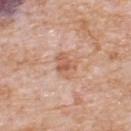Recorded during total-body skin imaging; not selected for excision or biopsy.
A male subject about 80 years old.
The lesion is on the upper back.
The recorded lesion diameter is about 3 mm.
This image is a 15 mm lesion crop taken from a total-body photograph.
The lesion-visualizer software estimated a shape-asymmetry score of about 0.2 (0 = symmetric). It also reported an average lesion color of about L≈60 a*≈22 b*≈32 (CIELAB) and a lesion–skin lightness drop of about 9. The software also gave border irregularity of about 2 on a 0–10 scale, a within-lesion color-variation index near 4/10, and peripheral color asymmetry of about 1.5.
This is a white-light tile.